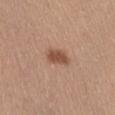{
  "biopsy_status": "not biopsied; imaged during a skin examination",
  "site": "front of the torso",
  "patient": {
    "sex": "female",
    "age_approx": 45
  },
  "lighting": "white-light",
  "lesion_size": {
    "long_diameter_mm_approx": 3.0
  },
  "image": {
    "source": "total-body photography crop",
    "field_of_view_mm": 15
  }
}